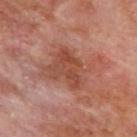Impression:
No biopsy was performed on this lesion — it was imaged during a full skin examination and was not determined to be concerning.
Clinical summary:
A 15 mm crop from a total-body photograph taken for skin-cancer surveillance. On the upper back. A male patient, approximately 70 years of age.